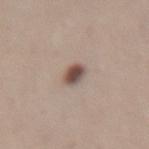This lesion was catalogued during total-body skin photography and was not selected for biopsy.
The tile uses white-light illumination.
Cropped from a total-body skin-imaging series; the visible field is about 15 mm.
The total-body-photography lesion software estimated a border-irregularity index near 1.5/10 and peripheral color asymmetry of about 2. And it measured a classifier nevus-likeness of about 100/100 and a detector confidence of about 100 out of 100 that the crop contains a lesion.
A female patient aged approximately 30.
Located on the lower back.
The recorded lesion diameter is about 2.5 mm.